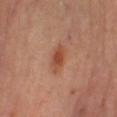<lesion>
<biopsy_status>not biopsied; imaged during a skin examination</biopsy_status>
<patient>
  <sex>female</sex>
  <age_approx>65</age_approx>
</patient>
<lesion_size>
  <long_diameter_mm_approx>3.5</long_diameter_mm_approx>
</lesion_size>
<site>left thigh</site>
<image>
  <source>total-body photography crop</source>
  <field_of_view_mm>15</field_of_view_mm>
</image>
<lighting>cross-polarized</lighting>
</lesion>